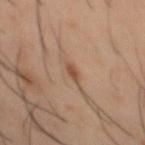notes: imaged on a skin check; not biopsied | image source: total-body-photography crop, ~15 mm field of view | subject: male, roughly 40 years of age | anatomic site: the mid back | diameter: about 3 mm | tile lighting: cross-polarized illumination.